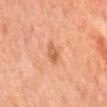biopsy_status: not biopsied; imaged during a skin examination
automated_metrics:
  area_mm2_approx: 3.5
  eccentricity: 0.8
  shape_asymmetry: 0.25
image:
  source: total-body photography crop
  field_of_view_mm: 15
patient:
  sex: male
  age_approx: 65
site: mid back
lighting: cross-polarized
lesion_size:
  long_diameter_mm_approx: 2.5